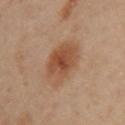Impression:
The lesion was photographed on a routine skin check and not biopsied; there is no pathology result.
Acquisition and patient details:
About 5 mm across. The patient is a female aged approximately 40. This is a cross-polarized tile. From the left upper arm. Automated tile analysis of the lesion measured a lesion area of about 15 mm². It also reported a mean CIELAB color near L≈50 a*≈21 b*≈33, roughly 10 lightness units darker than nearby skin, and a normalized lesion–skin contrast near 8. A region of skin cropped from a whole-body photographic capture, roughly 15 mm wide.